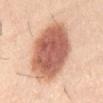* biopsy status — total-body-photography surveillance lesion; no biopsy
* lighting — white-light
* location — the front of the torso
* subject — male, approximately 40 years of age
* image source — ~15 mm crop, total-body skin-cancer survey